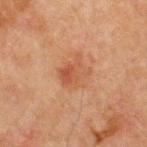<lesion>
<biopsy_status>not biopsied; imaged during a skin examination</biopsy_status>
<patient>
  <sex>male</sex>
  <age_approx>65</age_approx>
</patient>
<lesion_size>
  <long_diameter_mm_approx>4.0</long_diameter_mm_approx>
</lesion_size>
<site>chest</site>
<image>
  <source>total-body photography crop</source>
  <field_of_view_mm>15</field_of_view_mm>
</image>
<lighting>cross-polarized</lighting>
</lesion>